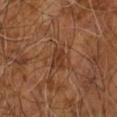biopsy_status: not biopsied; imaged during a skin examination
image:
  source: total-body photography crop
  field_of_view_mm: 15
patient:
  sex: male
  age_approx: 60
lighting: cross-polarized
automated_metrics:
  cielab_L: 34
  cielab_a: 21
  cielab_b: 30
  vs_skin_darker_L: 8.0
  vs_skin_contrast_norm: 7.0
  color_variation_0_10: 2.0
  peripheral_color_asymmetry: 0.5
  nevus_likeness_0_100: 15
site: right arm
lesion_size:
  long_diameter_mm_approx: 2.5